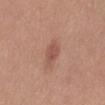Assessment: The lesion was tiled from a total-body skin photograph and was not biopsied. Background: This is a white-light tile. The lesion-visualizer software estimated internal color variation of about 1.5 on a 0–10 scale and peripheral color asymmetry of about 0.5. The software also gave a detector confidence of about 100 out of 100 that the crop contains a lesion. The lesion is located on the abdomen. A female subject aged 53–57. A 15 mm close-up tile from a total-body photography series done for melanoma screening. The lesion's longest dimension is about 2.5 mm.